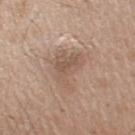body site: the right upper arm | patient: male, aged approximately 65 | image source: ~15 mm crop, total-body skin-cancer survey.